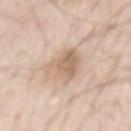{"biopsy_status": "not biopsied; imaged during a skin examination", "site": "mid back", "patient": {"sex": "male", "age_approx": 60}, "image": {"source": "total-body photography crop", "field_of_view_mm": 15}}